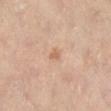A 15 mm crop from a total-body photograph taken for skin-cancer surveillance. A female subject, about 55 years old. The lesion is on the leg.